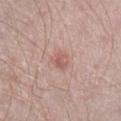<tbp_lesion>
<automated_metrics>
  <area_mm2_approx>4.5</area_mm2_approx>
  <eccentricity>0.6</eccentricity>
  <shape_asymmetry>0.25</shape_asymmetry>
  <lesion_detection_confidence_0_100>100</lesion_detection_confidence_0_100>
</automated_metrics>
<patient>
  <sex>male</sex>
  <age_approx>40</age_approx>
</patient>
<site>right lower leg</site>
<lighting>white-light</lighting>
<image>
  <source>total-body photography crop</source>
  <field_of_view_mm>15</field_of_view_mm>
</image>
<lesion_size>
  <long_diameter_mm_approx>2.5</long_diameter_mm_approx>
</lesion_size>
</tbp_lesion>